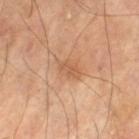Captured during whole-body skin photography for melanoma surveillance; the lesion was not biopsied. Automated tile analysis of the lesion measured an outline eccentricity of about 0.8 (0 = round, 1 = elongated) and a symmetry-axis asymmetry near 0.3. The tile uses cross-polarized illumination. A male patient, approximately 70 years of age. A 15 mm crop from a total-body photograph taken for skin-cancer surveillance. Located on the left thigh. Longest diameter approximately 3 mm.